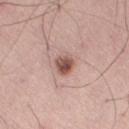follow-up = catalogued during a skin exam; not biopsied
size = about 3 mm
patient = male, approximately 70 years of age
body site = the leg
imaging modality = total-body-photography crop, ~15 mm field of view
image-analysis metrics = border irregularity of about 1.5 on a 0–10 scale, a within-lesion color-variation index near 6/10, and radial color variation of about 2.5; a detector confidence of about 100 out of 100 that the crop contains a lesion